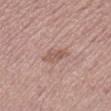No biopsy was performed on this lesion — it was imaged during a full skin examination and was not determined to be concerning. From the right lower leg. The subject is a female aged approximately 40. Imaged with white-light lighting. Longest diameter approximately 2.5 mm. This image is a 15 mm lesion crop taken from a total-body photograph.